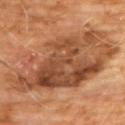- biopsy status: no biopsy performed (imaged during a skin exam)
- subject: male, in their 60s
- tile lighting: cross-polarized illumination
- anatomic site: the chest
- lesion diameter: ≈13 mm
- automated lesion analysis: a mean CIELAB color near L≈47 a*≈23 b*≈34, about 11 CIELAB-L* units darker than the surrounding skin, and a normalized lesion–skin contrast near 8.5
- image source: 15 mm crop, total-body photography